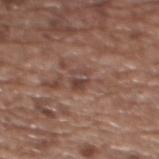Part of a total-body skin-imaging series; this lesion was reviewed on a skin check and was not flagged for biopsy.
Captured under white-light illumination.
Approximately 2.5 mm at its widest.
The lesion is on the upper back.
A lesion tile, about 15 mm wide, cut from a 3D total-body photograph.
A female patient in their mid- to late 70s.